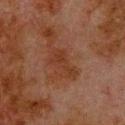follow-up = catalogued during a skin exam; not biopsied | image source = total-body-photography crop, ~15 mm field of view | lighting = cross-polarized | patient = male, aged 78 to 82 | diameter = ≈4 mm | location = the upper back.